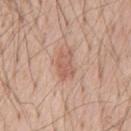A 15 mm close-up tile from a total-body photography series done for melanoma screening. Captured under white-light illumination. Measured at roughly 3.5 mm in maximum diameter. A male patient about 55 years old. The lesion is on the front of the torso.